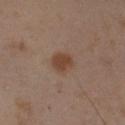<tbp_lesion>
  <biopsy_status>not biopsied; imaged during a skin examination</biopsy_status>
  <image>
    <source>total-body photography crop</source>
    <field_of_view_mm>15</field_of_view_mm>
  </image>
  <site>left upper arm</site>
  <patient>
    <sex>male</sex>
    <age_approx>55</age_approx>
  </patient>
  <lesion_size>
    <long_diameter_mm_approx>2.5</long_diameter_mm_approx>
  </lesion_size>
  <automated_metrics>
    <cielab_L>42</cielab_L>
    <cielab_a>18</cielab_a>
    <cielab_b>28</cielab_b>
    <vs_skin_darker_L>9.0</vs_skin_darker_L>
    <vs_skin_contrast_norm>8.0</vs_skin_contrast_norm>
  </automated_metrics>
</tbp_lesion>